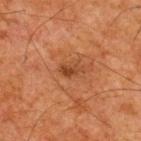Assessment:
This lesion was catalogued during total-body skin photography and was not selected for biopsy.
Clinical summary:
From the upper back. A male patient, in their mid-60s. Cropped from a whole-body photographic skin survey; the tile spans about 15 mm. Captured under cross-polarized illumination. The recorded lesion diameter is about 2.5 mm. Automated tile analysis of the lesion measured a lesion area of about 3 mm², a shape eccentricity near 0.85, and a shape-asymmetry score of about 0.4 (0 = symmetric). And it measured a border-irregularity rating of about 4/10, internal color variation of about 1 on a 0–10 scale, and peripheral color asymmetry of about 0.5. And it measured an automated nevus-likeness rating near 15 out of 100.